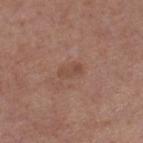Recorded during total-body skin imaging; not selected for excision or biopsy.
The tile uses white-light illumination.
About 2.5 mm across.
The lesion-visualizer software estimated a classifier nevus-likeness of about 0/100 and a lesion-detection confidence of about 100/100.
A male patient aged 53 to 57.
A 15 mm close-up tile from a total-body photography series done for melanoma screening.
From the right lower leg.